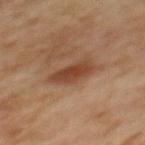This lesion was catalogued during total-body skin photography and was not selected for biopsy.
Located on the upper back.
A region of skin cropped from a whole-body photographic capture, roughly 15 mm wide.
A female patient about 60 years old.
About 4 mm across.
The tile uses cross-polarized illumination.